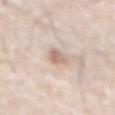biopsy status = catalogued during a skin exam; not biopsied
site = the abdomen
patient = male, approximately 80 years of age
image = ~15 mm crop, total-body skin-cancer survey